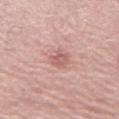Clinical impression: No biopsy was performed on this lesion — it was imaged during a full skin examination and was not determined to be concerning. Context: A female patient aged 68–72. The lesion is on the left thigh. This image is a 15 mm lesion crop taken from a total-body photograph.